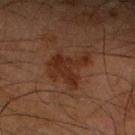Q: Was this lesion biopsied?
A: catalogued during a skin exam; not biopsied
Q: Lesion location?
A: the right forearm
Q: Who is the patient?
A: male, roughly 65 years of age
Q: How was this image acquired?
A: ~15 mm tile from a whole-body skin photo
Q: Lesion size?
A: about 4.5 mm